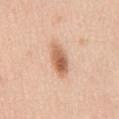follow-up: no biopsy performed (imaged during a skin exam)
lesion diameter: ~4.5 mm (longest diameter)
tile lighting: white-light illumination
image: ~15 mm crop, total-body skin-cancer survey
subject: male, aged 48 to 52
body site: the abdomen